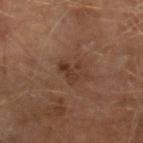• patient · male, aged approximately 65
• site · the right lower leg
• illumination · cross-polarized illumination
• image source · ~15 mm crop, total-body skin-cancer survey
• diameter · ≈3.5 mm